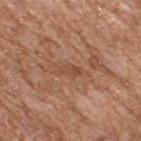follow-up: no biopsy performed (imaged during a skin exam)